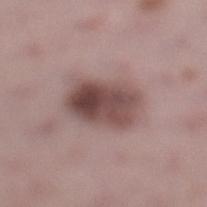The patient is a female aged approximately 45. Cropped from a whole-body photographic skin survey; the tile spans about 15 mm. The total-body-photography lesion software estimated a lesion–skin lightness drop of about 14 and a normalized lesion–skin contrast near 10. The lesion is on the leg. Approximately 6 mm at its widest. This is a white-light tile.